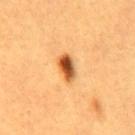Assessment:
This lesion was catalogued during total-body skin photography and was not selected for biopsy.
Image and clinical context:
The subject is a male roughly 60 years of age. A 15 mm crop from a total-body photograph taken for skin-cancer surveillance. Longest diameter approximately 3 mm. From the abdomen. The tile uses cross-polarized illumination.